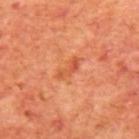Part of a total-body skin-imaging series; this lesion was reviewed on a skin check and was not flagged for biopsy.
Measured at roughly 3.5 mm in maximum diameter.
The lesion-visualizer software estimated a footprint of about 4 mm², an outline eccentricity of about 0.9 (0 = round, 1 = elongated), and a shape-asymmetry score of about 0.4 (0 = symmetric). The analysis additionally found a classifier nevus-likeness of about 0/100 and a lesion-detection confidence of about 100/100.
A male patient aged 68–72.
A close-up tile cropped from a whole-body skin photograph, about 15 mm across.
Located on the mid back.
This is a cross-polarized tile.